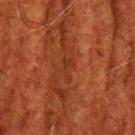workup = catalogued during a skin exam; not biopsied
subject = male, in their 60s
image source = 15 mm crop, total-body photography
anatomic site = the head or neck